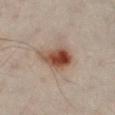workup: imaged on a skin check; not biopsied
patient: male, aged 48 to 52
lighting: cross-polarized
diameter: about 5.5 mm
automated lesion analysis: a footprint of about 11 mm², an outline eccentricity of about 0.85 (0 = round, 1 = elongated), and two-axis asymmetry of about 0.25; a mean CIELAB color near L≈39 a*≈17 b*≈24, about 12 CIELAB-L* units darker than the surrounding skin, and a normalized border contrast of about 11; a border-irregularity rating of about 3/10; a nevus-likeness score of about 100/100 and a detector confidence of about 100 out of 100 that the crop contains a lesion
site: the left leg
imaging modality: total-body-photography crop, ~15 mm field of view